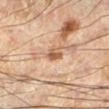site: the leg
TBP lesion metrics: a nevus-likeness score of about 15/100 and a lesion-detection confidence of about 100/100
diameter: about 2 mm
subject: male, aged approximately 60
lighting: cross-polarized
imaging modality: 15 mm crop, total-body photography